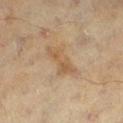Captured during whole-body skin photography for melanoma surveillance; the lesion was not biopsied.
The subject is a female aged around 60.
This is a cross-polarized tile.
Located on the leg.
A lesion tile, about 15 mm wide, cut from a 3D total-body photograph.
The recorded lesion diameter is about 4.5 mm.